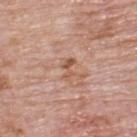No biopsy was performed on this lesion — it was imaged during a full skin examination and was not determined to be concerning. Located on the upper back. About 2.5 mm across. The subject is a male about 75 years old. A 15 mm crop from a total-body photograph taken for skin-cancer surveillance. Automated tile analysis of the lesion measured an area of roughly 3 mm², an eccentricity of roughly 0.85, and a shape-asymmetry score of about 0.45 (0 = symmetric). The software also gave an average lesion color of about L≈55 a*≈22 b*≈30 (CIELAB), a lesion–skin lightness drop of about 9, and a normalized lesion–skin contrast near 6.5. The analysis additionally found a detector confidence of about 100 out of 100 that the crop contains a lesion. Imaged with white-light lighting.